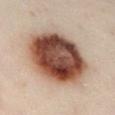Recorded during total-body skin imaging; not selected for excision or biopsy.
Located on the left lower leg.
A close-up tile cropped from a whole-body skin photograph, about 15 mm across.
A male patient roughly 50 years of age.